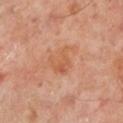Clinical impression:
The lesion was photographed on a routine skin check and not biopsied; there is no pathology result.
Image and clinical context:
The lesion is located on the left lower leg. A 15 mm crop from a total-body photograph taken for skin-cancer surveillance. The tile uses cross-polarized illumination. The patient is a male aged around 50. Automated image analysis of the tile measured a lesion area of about 8 mm², an eccentricity of roughly 0.55, and two-axis asymmetry of about 0.4. The software also gave an automated nevus-likeness rating near 0 out of 100. Longest diameter approximately 4 mm.